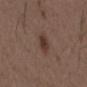Notes:
- biopsy status — total-body-photography surveillance lesion; no biopsy
- diameter — ~3 mm (longest diameter)
- location — the chest
- patient — male, aged 48 to 52
- acquisition — total-body-photography crop, ~15 mm field of view